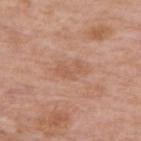<record>
  <biopsy_status>not biopsied; imaged during a skin examination</biopsy_status>
  <site>upper back</site>
  <patient>
    <sex>male</sex>
    <age_approx>75</age_approx>
  </patient>
  <image>
    <source>total-body photography crop</source>
    <field_of_view_mm>15</field_of_view_mm>
  </image>
  <lighting>white-light</lighting>
</record>